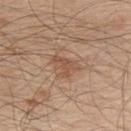workup: imaged on a skin check; not biopsied
subject: male, aged approximately 50
anatomic site: the upper back
image: total-body-photography crop, ~15 mm field of view